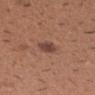{"biopsy_status": "not biopsied; imaged during a skin examination", "image": {"source": "total-body photography crop", "field_of_view_mm": 15}, "site": "arm", "automated_metrics": {"area_mm2_approx": 4.5, "eccentricity": 0.55, "border_irregularity_0_10": 2.0, "color_variation_0_10": 2.0, "peripheral_color_asymmetry": 0.5, "nevus_likeness_0_100": 65, "lesion_detection_confidence_0_100": 100}, "patient": {"sex": "male", "age_approx": 40}, "lighting": "white-light"}